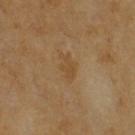notes: no biopsy performed (imaged during a skin exam)
patient: male, aged 63–67
acquisition: total-body-photography crop, ~15 mm field of view
lesion diameter: about 3.5 mm
TBP lesion metrics: a lesion area of about 4.5 mm², an eccentricity of roughly 0.85, and a symmetry-axis asymmetry near 0.35; lesion-presence confidence of about 100/100
anatomic site: the right upper arm